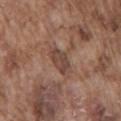workup: total-body-photography surveillance lesion; no biopsy
lighting: white-light illumination
subject: male, in their mid- to late 70s
image: ~15 mm crop, total-body skin-cancer survey
automated lesion analysis: an area of roughly 5.5 mm², a shape eccentricity near 0.8, and a symmetry-axis asymmetry near 0.25; a within-lesion color-variation index near 3/10 and peripheral color asymmetry of about 1
body site: the mid back
size: about 3.5 mm